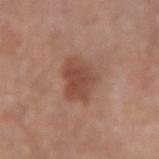follow-up: catalogued during a skin exam; not biopsied
subject: male, roughly 55 years of age
site: the arm
size: ≈4.5 mm
image: total-body-photography crop, ~15 mm field of view
image-analysis metrics: an average lesion color of about L≈48 a*≈22 b*≈28 (CIELAB) and a lesion-to-skin contrast of about 7 (normalized; higher = more distinct)